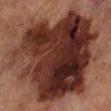follow-up: total-body-photography surveillance lesion; no biopsy
image source: ~15 mm crop, total-body skin-cancer survey
site: the left lower leg
diameter: ~14 mm (longest diameter)
TBP lesion metrics: a lesion area of about 110 mm², an outline eccentricity of about 0.45 (0 = round, 1 = elongated), and a symmetry-axis asymmetry near 0.25; a border-irregularity index near 4/10, a color-variation rating of about 9.5/10, and peripheral color asymmetry of about 3.5
patient: male, aged approximately 70
lighting: cross-polarized